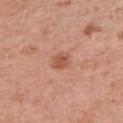workup: imaged on a skin check; not biopsied | image-analysis metrics: a mean CIELAB color near L≈55 a*≈25 b*≈32 and a normalized lesion–skin contrast near 6.5; a border-irregularity index near 2.5/10, a color-variation rating of about 2.5/10, and radial color variation of about 0.5; a classifier nevus-likeness of about 75/100 and a detector confidence of about 100 out of 100 that the crop contains a lesion | site: the right upper arm | lighting: white-light illumination | subject: female, approximately 40 years of age | image: ~15 mm tile from a whole-body skin photo | lesion size: ~2.5 mm (longest diameter).